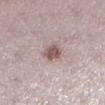Impression:
Captured during whole-body skin photography for melanoma surveillance; the lesion was not biopsied.
Clinical summary:
The subject is a female approximately 30 years of age. From the left lower leg. A lesion tile, about 15 mm wide, cut from a 3D total-body photograph.